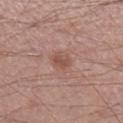Clinical impression: The lesion was tiled from a total-body skin photograph and was not biopsied. Context: Cropped from a total-body skin-imaging series; the visible field is about 15 mm. A male subject in their mid- to late 50s. Imaged with white-light lighting. Approximately 2.5 mm at its widest. The total-body-photography lesion software estimated about 8 CIELAB-L* units darker than the surrounding skin and a normalized lesion–skin contrast near 6. Located on the right lower leg.